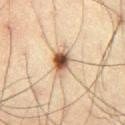{
  "biopsy_status": "not biopsied; imaged during a skin examination",
  "patient": {
    "sex": "male",
    "age_approx": 35
  },
  "lighting": "cross-polarized",
  "automated_metrics": {
    "border_irregularity_0_10": 2.5,
    "color_variation_0_10": 9.0,
    "peripheral_color_asymmetry": 2.5
  },
  "image": {
    "source": "total-body photography crop",
    "field_of_view_mm": 15
  },
  "site": "leg",
  "lesion_size": {
    "long_diameter_mm_approx": 4.0
  }
}